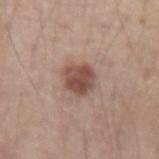Recorded during total-body skin imaging; not selected for excision or biopsy. Cropped from a total-body skin-imaging series; the visible field is about 15 mm. Approximately 3.5 mm at its widest. The patient is a male approximately 45 years of age. The tile uses white-light illumination. An algorithmic analysis of the crop reported a color-variation rating of about 4/10 and radial color variation of about 1.5. On the left forearm.